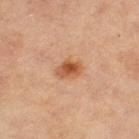follow-up: total-body-photography surveillance lesion; no biopsy
illumination: cross-polarized
anatomic site: the left thigh
patient: female, about 70 years old
image source: ~15 mm crop, total-body skin-cancer survey
size: about 3 mm